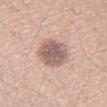follow-up: no biopsy performed (imaged during a skin exam) | lesion diameter: ~4.5 mm (longest diameter) | image: 15 mm crop, total-body photography | location: the right forearm | image-analysis metrics: an area of roughly 13 mm², an eccentricity of roughly 0.6, and a shape-asymmetry score of about 0.1 (0 = symmetric); border irregularity of about 1.5 on a 0–10 scale and radial color variation of about 1 | patient: female, about 35 years old | illumination: white-light.